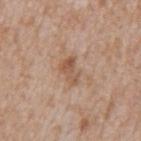Case summary:
- workup: catalogued during a skin exam; not biopsied
- image source: ~15 mm tile from a whole-body skin photo
- subject: male, aged around 65
- size: ≈3 mm
- site: the mid back
- TBP lesion metrics: an area of roughly 5.5 mm² and a shape eccentricity near 0.75; a nevus-likeness score of about 0/100 and a lesion-detection confidence of about 100/100